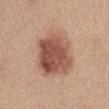– notes · no biopsy performed (imaged during a skin exam)
– site · the abdomen
– automated lesion analysis · a border-irregularity rating of about 2.5/10, internal color variation of about 7 on a 0–10 scale, and radial color variation of about 2.5; a nevus-likeness score of about 100/100 and a detector confidence of about 100 out of 100 that the crop contains a lesion
– diameter · about 6.5 mm
– tile lighting · white-light
– subject · female, aged approximately 45
– image source · ~15 mm crop, total-body skin-cancer survey